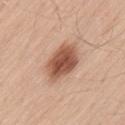| field | value |
|---|---|
| biopsy status | total-body-photography surveillance lesion; no biopsy |
| patient | male, approximately 55 years of age |
| image | 15 mm crop, total-body photography |
| site | the lower back |
| lighting | white-light |
| lesion size | ~5 mm (longest diameter) |